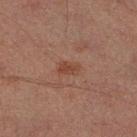The lesion was photographed on a routine skin check and not biopsied; there is no pathology result. A male subject about 30 years old. A lesion tile, about 15 mm wide, cut from a 3D total-body photograph. Located on the right lower leg.